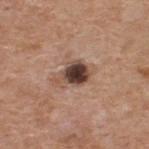A male patient, roughly 60 years of age.
Captured under white-light illumination.
A close-up tile cropped from a whole-body skin photograph, about 15 mm across.
The lesion is on the upper back.